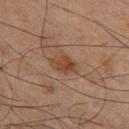Assessment: The lesion was tiled from a total-body skin photograph and was not biopsied. Clinical summary: A male subject, about 65 years old. From the left thigh. A 15 mm crop from a total-body photograph taken for skin-cancer surveillance. Captured under cross-polarized illumination. The total-body-photography lesion software estimated an outline eccentricity of about 0.65 (0 = round, 1 = elongated) and a symmetry-axis asymmetry near 0.2. The software also gave a mean CIELAB color near L≈34 a*≈17 b*≈26 and a normalized border contrast of about 7.5. It also reported border irregularity of about 2 on a 0–10 scale and internal color variation of about 2.5 on a 0–10 scale. It also reported a classifier nevus-likeness of about 60/100. The lesion's longest dimension is about 3 mm.